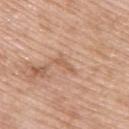Imaged during a routine full-body skin examination; the lesion was not biopsied and no histopathology is available. Longest diameter approximately 2.5 mm. The tile uses white-light illumination. From the back. A female patient in their mid- to late 50s. A region of skin cropped from a whole-body photographic capture, roughly 15 mm wide.